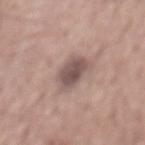This lesion was catalogued during total-body skin photography and was not selected for biopsy.
Located on the mid back.
This image is a 15 mm lesion crop taken from a total-body photograph.
A male subject aged 53 to 57.